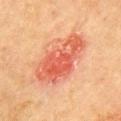A 15 mm close-up extracted from a 3D total-body photography capture. The tile uses cross-polarized illumination. The subject is a female aged 78 to 82. On the arm. Measured at roughly 7 mm in maximum diameter. Automated tile analysis of the lesion measured an eccentricity of roughly 0.85. It also reported an automated nevus-likeness rating near 30 out of 100.